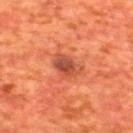notes = total-body-photography surveillance lesion; no biopsy
patient = male, approximately 65 years of age
illumination = cross-polarized
image source = ~15 mm tile from a whole-body skin photo
body site = the upper back